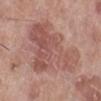This lesion was catalogued during total-body skin photography and was not selected for biopsy. This image is a 15 mm lesion crop taken from a total-body photograph. The tile uses white-light illumination. A female patient aged around 70. The lesion is on the left lower leg.